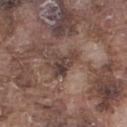Recorded during total-body skin imaging; not selected for excision or biopsy.
The lesion is on the right thigh.
A 15 mm close-up tile from a total-body photography series done for melanoma screening.
The tile uses white-light illumination.
A male patient, aged 73 to 77.